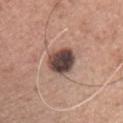Part of a total-body skin-imaging series; this lesion was reviewed on a skin check and was not flagged for biopsy.
The total-body-photography lesion software estimated a lesion area of about 10 mm² and a shape-asymmetry score of about 0.2 (0 = symmetric). The analysis additionally found a lesion color around L≈44 a*≈17 b*≈21 in CIELAB and a normalized lesion–skin contrast near 14. The analysis additionally found a border-irregularity rating of about 2/10 and a peripheral color-asymmetry measure near 2. The software also gave a lesion-detection confidence of about 100/100.
Cropped from a whole-body photographic skin survey; the tile spans about 15 mm.
About 4 mm across.
Captured under white-light illumination.
On the front of the torso.
The subject is a male about 45 years old.